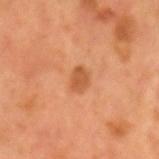Q: Automated lesion metrics?
A: a mean CIELAB color near L≈51 a*≈26 b*≈38 and a lesion-to-skin contrast of about 6.5 (normalized; higher = more distinct); a border-irregularity rating of about 1.5/10, internal color variation of about 2 on a 0–10 scale, and radial color variation of about 0.5
Q: What is the imaging modality?
A: 15 mm crop, total-body photography
Q: How was the tile lit?
A: cross-polarized illumination
Q: What is the anatomic site?
A: the head or neck
Q: Who is the patient?
A: male, in their mid-50s